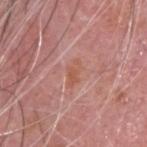Impression:
Imaged during a routine full-body skin examination; the lesion was not biopsied and no histopathology is available.
Background:
Cropped from a total-body skin-imaging series; the visible field is about 15 mm. A male subject, aged 73 to 77. The lesion is located on the head or neck. Measured at roughly 3 mm in maximum diameter. Captured under white-light illumination.